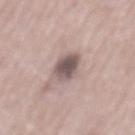Clinical impression:
No biopsy was performed on this lesion — it was imaged during a full skin examination and was not determined to be concerning.
Clinical summary:
Cropped from a whole-body photographic skin survey; the tile spans about 15 mm. On the mid back. Approximately 4 mm at its widest. A male subject aged around 65. Automated image analysis of the tile measured an average lesion color of about L≈53 a*≈15 b*≈17 (CIELAB), a lesion–skin lightness drop of about 15, and a lesion-to-skin contrast of about 10.5 (normalized; higher = more distinct). It also reported border irregularity of about 1.5 on a 0–10 scale, internal color variation of about 4 on a 0–10 scale, and radial color variation of about 1. It also reported an automated nevus-likeness rating near 45 out of 100 and a lesion-detection confidence of about 100/100. This is a white-light tile.